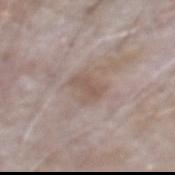Recorded during total-body skin imaging; not selected for excision or biopsy.
A 15 mm close-up tile from a total-body photography series done for melanoma screening.
The subject is a male aged around 65.
On the abdomen.